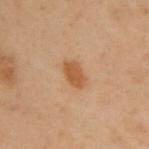Part of a total-body skin-imaging series; this lesion was reviewed on a skin check and was not flagged for biopsy.
Imaged with cross-polarized lighting.
On the upper back.
A 15 mm close-up extracted from a 3D total-body photography capture.
The recorded lesion diameter is about 3.5 mm.
A female patient aged around 60.